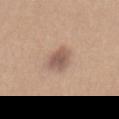workup: imaged on a skin check; not biopsied
subject: female, aged around 40
anatomic site: the front of the torso
lighting: white-light
lesion diameter: ~4 mm (longest diameter)
acquisition: ~15 mm crop, total-body skin-cancer survey
TBP lesion metrics: an area of roughly 7 mm², an eccentricity of roughly 0.85, and a shape-asymmetry score of about 0.25 (0 = symmetric); a mean CIELAB color near L≈56 a*≈17 b*≈26, a lesion–skin lightness drop of about 11, and a normalized border contrast of about 8